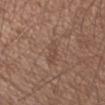Q: What is the anatomic site?
A: the left upper arm
Q: Who is the patient?
A: male, aged approximately 55
Q: How was this image acquired?
A: total-body-photography crop, ~15 mm field of view